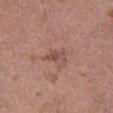notes: no biopsy performed (imaged during a skin exam) | lesion diameter: ~3 mm (longest diameter) | lighting: white-light | subject: female, aged 63 to 67 | anatomic site: the right thigh | acquisition: ~15 mm crop, total-body skin-cancer survey.